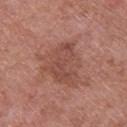Longest diameter approximately 5 mm. This image is a 15 mm lesion crop taken from a total-body photograph. A female subject about 40 years old. The lesion-visualizer software estimated border irregularity of about 6.5 on a 0–10 scale and peripheral color asymmetry of about 1. The analysis additionally found a nevus-likeness score of about 5/100 and lesion-presence confidence of about 100/100. Imaged with white-light lighting. On the upper back.